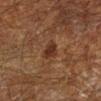Impression:
This lesion was catalogued during total-body skin photography and was not selected for biopsy.
Background:
Approximately 2.5 mm at its widest. A 15 mm crop from a total-body photograph taken for skin-cancer surveillance. This is a cross-polarized tile. The patient is a male in their 60s. The total-body-photography lesion software estimated a footprint of about 4 mm², an outline eccentricity of about 0.65 (0 = round, 1 = elongated), and a shape-asymmetry score of about 0.3 (0 = symmetric). The software also gave a lesion color around L≈24 a*≈17 b*≈23 in CIELAB, roughly 7 lightness units darker than nearby skin, and a normalized border contrast of about 8.5. And it measured a border-irregularity index near 2.5/10 and a color-variation rating of about 1.5/10. The analysis additionally found a nevus-likeness score of about 80/100 and lesion-presence confidence of about 100/100. The lesion is on the leg.